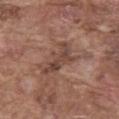Assessment: The lesion was tiled from a total-body skin photograph and was not biopsied. Clinical summary: Captured under white-light illumination. Located on the abdomen. A close-up tile cropped from a whole-body skin photograph, about 15 mm across. Measured at roughly 5 mm in maximum diameter. The subject is a male in their mid- to late 70s.